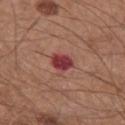* location · the front of the torso
* subject · male, aged around 65
* lesion diameter · ≈3 mm
* lighting · white-light
* image · total-body-photography crop, ~15 mm field of view